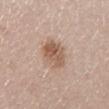<case>
  <biopsy_status>not biopsied; imaged during a skin examination</biopsy_status>
  <patient>
    <sex>female</sex>
    <age_approx>40</age_approx>
  </patient>
  <site>right lower leg</site>
  <image>
    <source>total-body photography crop</source>
    <field_of_view_mm>15</field_of_view_mm>
  </image>
  <lesion_size>
    <long_diameter_mm_approx>3.5</long_diameter_mm_approx>
  </lesion_size>
</case>